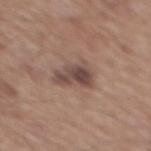biopsy status: catalogued during a skin exam; not biopsied
image: total-body-photography crop, ~15 mm field of view
site: the mid back
lighting: white-light illumination
patient: male, in their mid- to late 60s